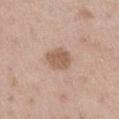Captured during whole-body skin photography for melanoma surveillance; the lesion was not biopsied.
Longest diameter approximately 3 mm.
A male subject, aged around 50.
From the left thigh.
A 15 mm close-up tile from a total-body photography series done for melanoma screening.
The total-body-photography lesion software estimated an average lesion color of about L≈58 a*≈18 b*≈29 (CIELAB) and a normalized border contrast of about 7.5.